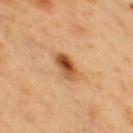Q: Is there a histopathology result?
A: total-body-photography surveillance lesion; no biopsy
Q: Lesion location?
A: the chest
Q: How was this image acquired?
A: ~15 mm crop, total-body skin-cancer survey
Q: What is the lesion's diameter?
A: ~3.5 mm (longest diameter)
Q: How was the tile lit?
A: cross-polarized
Q: Patient demographics?
A: male, aged 53 to 57